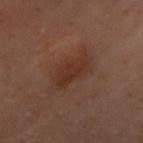notes: total-body-photography surveillance lesion; no biopsy
anatomic site: the left arm
imaging modality: 15 mm crop, total-body photography
subject: female, aged 58 to 62
TBP lesion metrics: a lesion area of about 10 mm², an outline eccentricity of about 0.9 (0 = round, 1 = elongated), and a symmetry-axis asymmetry near 0.3; a mean CIELAB color near L≈28 a*≈18 b*≈23, a lesion–skin lightness drop of about 6, and a normalized border contrast of about 7; border irregularity of about 3.5 on a 0–10 scale, internal color variation of about 2.5 on a 0–10 scale, and radial color variation of about 1; an automated nevus-likeness rating near 40 out of 100 and a detector confidence of about 100 out of 100 that the crop contains a lesion